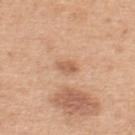This lesion was catalogued during total-body skin photography and was not selected for biopsy. Imaged with white-light lighting. The recorded lesion diameter is about 2.5 mm. A region of skin cropped from a whole-body photographic capture, roughly 15 mm wide. A male subject aged 48–52. The lesion is on the back.